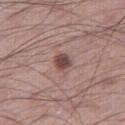Impression:
This lesion was catalogued during total-body skin photography and was not selected for biopsy.
Acquisition and patient details:
A male patient, aged approximately 60. A region of skin cropped from a whole-body photographic capture, roughly 15 mm wide. The tile uses white-light illumination. Located on the right thigh. Longest diameter approximately 2.5 mm. Automated tile analysis of the lesion measured an area of roughly 4.5 mm² and a shape eccentricity near 0.6. It also reported a lesion color around L≈47 a*≈19 b*≈20 in CIELAB. And it measured a border-irregularity index near 1.5/10 and radial color variation of about 1.5.